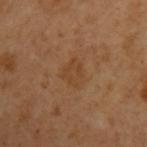follow-up: catalogued during a skin exam; not biopsied
lesion size: ≈3 mm
anatomic site: the left upper arm
patient: male, roughly 50 years of age
imaging modality: 15 mm crop, total-body photography